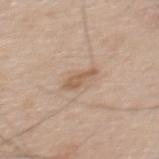Q: Was a biopsy performed?
A: catalogued during a skin exam; not biopsied
Q: How was this image acquired?
A: total-body-photography crop, ~15 mm field of view
Q: What is the anatomic site?
A: the upper back
Q: Illumination type?
A: white-light
Q: What is the lesion's diameter?
A: ≈3.5 mm
Q: What are the patient's age and sex?
A: male, about 65 years old